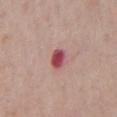Q: Was this lesion biopsied?
A: catalogued during a skin exam; not biopsied
Q: What is the anatomic site?
A: the front of the torso
Q: How was the tile lit?
A: white-light illumination
Q: What is the lesion's diameter?
A: ≈2.5 mm
Q: Patient demographics?
A: male, about 60 years old
Q: What kind of image is this?
A: total-body-photography crop, ~15 mm field of view